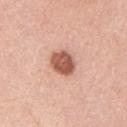Captured during whole-body skin photography for melanoma surveillance; the lesion was not biopsied. Automated tile analysis of the lesion measured a shape eccentricity near 0.45. It also reported a lesion color around L≈56 a*≈25 b*≈30 in CIELAB and a normalized border contrast of about 10.5. The analysis additionally found a border-irregularity index near 1.5/10 and a color-variation rating of about 3.5/10. A roughly 15 mm field-of-view crop from a total-body skin photograph. The subject is a male aged 38 to 42. Approximately 3 mm at its widest. Captured under white-light illumination. Located on the left upper arm.